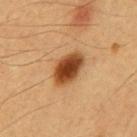biopsy_status: not biopsied; imaged during a skin examination
patient:
  sex: male
  age_approx: 60
image:
  source: total-body photography crop
  field_of_view_mm: 15
automated_metrics:
  cielab_L: 43
  cielab_a: 22
  cielab_b: 36
  vs_skin_darker_L: 17.0
  vs_skin_contrast_norm: 12.5
  color_variation_0_10: 5.0
  peripheral_color_asymmetry: 1.5
  nevus_likeness_0_100: 100
  lesion_detection_confidence_0_100: 100
site: front of the torso
lesion_size:
  long_diameter_mm_approx: 4.5